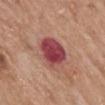{"biopsy_status": "not biopsied; imaged during a skin examination", "lighting": "white-light", "lesion_size": {"long_diameter_mm_approx": 4.0}, "image": {"source": "total-body photography crop", "field_of_view_mm": 15}, "patient": {"sex": "female", "age_approx": 75}, "site": "back"}